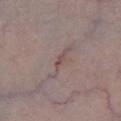| field | value |
|---|---|
| biopsy status | catalogued during a skin exam; not biopsied |
| anatomic site | the left lower leg |
| imaging modality | ~15 mm tile from a whole-body skin photo |
| subject | male, roughly 80 years of age |
| tile lighting | white-light |
| lesion diameter | about 1.5 mm |